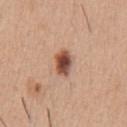Q: Was a biopsy performed?
A: imaged on a skin check; not biopsied
Q: Who is the patient?
A: male, in their 40s
Q: How was the tile lit?
A: white-light illumination
Q: Automated lesion metrics?
A: an area of roughly 6 mm² and a symmetry-axis asymmetry near 0.2; a color-variation rating of about 8/10 and radial color variation of about 2.5; an automated nevus-likeness rating near 100 out of 100
Q: Lesion location?
A: the front of the torso
Q: What is the lesion's diameter?
A: ~3.5 mm (longest diameter)
Q: How was this image acquired?
A: ~15 mm crop, total-body skin-cancer survey